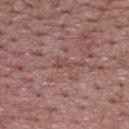Q: Was this lesion biopsied?
A: catalogued during a skin exam; not biopsied
Q: Automated lesion metrics?
A: an average lesion color of about L≈47 a*≈21 b*≈22 (CIELAB) and a normalized lesion–skin contrast near 5; a border-irregularity index near 5.5/10, a within-lesion color-variation index near 0/10, and radial color variation of about 0; a nevus-likeness score of about 0/100 and lesion-presence confidence of about 65/100
Q: Lesion size?
A: about 2.5 mm
Q: Illumination type?
A: white-light
Q: Lesion location?
A: the back
Q: Who is the patient?
A: male, aged 68 to 72
Q: How was this image acquired?
A: total-body-photography crop, ~15 mm field of view